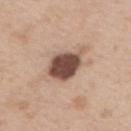The lesion was photographed on a routine skin check and not biopsied; there is no pathology result. A region of skin cropped from a whole-body photographic capture, roughly 15 mm wide. The subject is a male aged around 40. Measured at roughly 4.5 mm in maximum diameter. Automated tile analysis of the lesion measured an eccentricity of roughly 0.7 and a symmetry-axis asymmetry near 0.2. The software also gave a mean CIELAB color near L≈48 a*≈19 b*≈24 and about 20 CIELAB-L* units darker than the surrounding skin. The analysis additionally found an automated nevus-likeness rating near 75 out of 100. The lesion is located on the upper back. Imaged with white-light lighting.